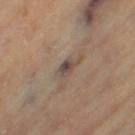{
  "biopsy_status": "not biopsied; imaged during a skin examination",
  "image": {
    "source": "total-body photography crop",
    "field_of_view_mm": 15
  },
  "site": "left thigh",
  "automated_metrics": {
    "area_mm2_approx": 3.5,
    "eccentricity": 0.8,
    "color_variation_0_10": 5.0,
    "nevus_likeness_0_100": 0
  },
  "patient": {
    "sex": "female",
    "age_approx": 70
  },
  "lesion_size": {
    "long_diameter_mm_approx": 2.5
  },
  "lighting": "cross-polarized"
}